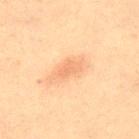The lesion was photographed on a routine skin check and not biopsied; there is no pathology result. A male subject, approximately 60 years of age. Imaged with cross-polarized lighting. Automated image analysis of the tile measured a border-irregularity rating of about 3.5/10 and peripheral color asymmetry of about 0.5. Cropped from a total-body skin-imaging series; the visible field is about 15 mm. The lesion is on the back.